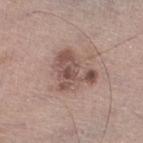Imaged during a routine full-body skin examination; the lesion was not biopsied and no histopathology is available.
The lesion is on the left lower leg.
This image is a 15 mm lesion crop taken from a total-body photograph.
Imaged with white-light lighting.
A male patient, aged around 70.
Approximately 5 mm at its widest.